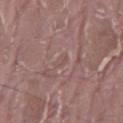The lesion was tiled from a total-body skin photograph and was not biopsied. The subject is a male approximately 40 years of age. Approximately 2.5 mm at its widest. The lesion is on the mid back. Automated image analysis of the tile measured an area of roughly 2 mm², an outline eccentricity of about 0.9 (0 = round, 1 = elongated), and a symmetry-axis asymmetry near 0.4. And it measured an average lesion color of about L≈51 a*≈18 b*≈21 (CIELAB), roughly 4 lightness units darker than nearby skin, and a normalized lesion–skin contrast near 3. It also reported a border-irregularity rating of about 4.5/10, a within-lesion color-variation index near 0/10, and a peripheral color-asymmetry measure near 0. The software also gave a classifier nevus-likeness of about 0/100 and lesion-presence confidence of about 0/100. A 15 mm close-up tile from a total-body photography series done for melanoma screening.